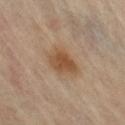This lesion was catalogued during total-body skin photography and was not selected for biopsy. A lesion tile, about 15 mm wide, cut from a 3D total-body photograph. The lesion is located on the right lower leg. The tile uses cross-polarized illumination. A female patient in their mid-60s.